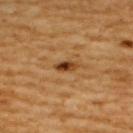{
  "biopsy_status": "not biopsied; imaged during a skin examination",
  "lesion_size": {
    "long_diameter_mm_approx": 3.0
  },
  "lighting": "cross-polarized",
  "automated_metrics": {
    "border_irregularity_0_10": 3.0,
    "peripheral_color_asymmetry": 3.0,
    "nevus_likeness_0_100": 100,
    "lesion_detection_confidence_0_100": 100
  },
  "patient": {
    "sex": "male",
    "age_approx": 60
  },
  "image": {
    "source": "total-body photography crop",
    "field_of_view_mm": 15
  },
  "site": "back"
}